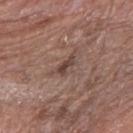Q: Was a biopsy performed?
A: catalogued during a skin exam; not biopsied
Q: Where on the body is the lesion?
A: the right forearm
Q: How was the tile lit?
A: white-light
Q: How large is the lesion?
A: about 2.5 mm
Q: What are the patient's age and sex?
A: female, roughly 75 years of age
Q: What is the imaging modality?
A: ~15 mm crop, total-body skin-cancer survey